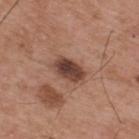- biopsy status: catalogued during a skin exam; not biopsied
- image-analysis metrics: a lesion area of about 7 mm², an outline eccentricity of about 0.8 (0 = round, 1 = elongated), and a shape-asymmetry score of about 0.15 (0 = symmetric)
- illumination: white-light
- patient: male, approximately 55 years of age
- acquisition: ~15 mm tile from a whole-body skin photo
- lesion diameter: ~4 mm (longest diameter)
- site: the upper back